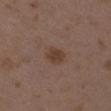Findings:
- anatomic site — the left upper arm
- lighting — white-light
- patient — female, aged approximately 35
- TBP lesion metrics — an outline eccentricity of about 0.65 (0 = round, 1 = elongated) and two-axis asymmetry of about 0.15; a lesion color around L≈39 a*≈17 b*≈26 in CIELAB, a lesion–skin lightness drop of about 7, and a normalized border contrast of about 7.5
- imaging modality — 15 mm crop, total-body photography
- size — ≈2.5 mm